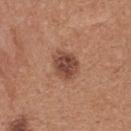No biopsy was performed on this lesion — it was imaged during a full skin examination and was not determined to be concerning.
Located on the upper back.
Cropped from a whole-body photographic skin survey; the tile spans about 15 mm.
Approximately 3.5 mm at its widest.
A female subject, about 40 years old.
This is a white-light tile.